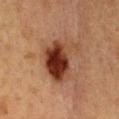Findings:
- notes — imaged on a skin check; not biopsied
- illumination — cross-polarized illumination
- site — the chest
- size — about 5.5 mm
- image source — ~15 mm crop, total-body skin-cancer survey
- patient — female, roughly 40 years of age